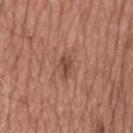Captured during whole-body skin photography for melanoma surveillance; the lesion was not biopsied. The tile uses white-light illumination. A male patient, roughly 55 years of age. This image is a 15 mm lesion crop taken from a total-body photograph. The lesion-visualizer software estimated a color-variation rating of about 1/10 and radial color variation of about 0.5. On the head or neck. Longest diameter approximately 2.5 mm.